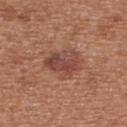<case>
<biopsy_status>not biopsied; imaged during a skin examination</biopsy_status>
<site>upper back</site>
<image>
  <source>total-body photography crop</source>
  <field_of_view_mm>15</field_of_view_mm>
</image>
<lighting>white-light</lighting>
<patient>
  <sex>female</sex>
  <age_approx>40</age_approx>
</patient>
</case>